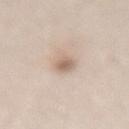image: ~15 mm crop, total-body skin-cancer survey
location: the lower back
patient: female, approximately 75 years of age